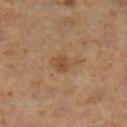Assessment: This lesion was catalogued during total-body skin photography and was not selected for biopsy. Acquisition and patient details: Automated tile analysis of the lesion measured an eccentricity of roughly 0.8 and a shape-asymmetry score of about 0.35 (0 = symmetric). And it measured a border-irregularity index near 3.5/10, internal color variation of about 2 on a 0–10 scale, and radial color variation of about 0.5. It also reported a lesion-detection confidence of about 100/100. Captured under cross-polarized illumination. A female patient, aged 68 to 72. The lesion is located on the left lower leg. A 15 mm close-up tile from a total-body photography series done for melanoma screening. Measured at roughly 3.5 mm in maximum diameter.